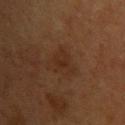Part of a total-body skin-imaging series; this lesion was reviewed on a skin check and was not flagged for biopsy.
A lesion tile, about 15 mm wide, cut from a 3D total-body photograph.
From the upper back.
The subject is a female aged around 40.